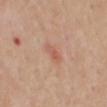A male patient aged approximately 65.
On the mid back.
A close-up tile cropped from a whole-body skin photograph, about 15 mm across.